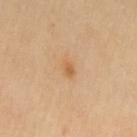On the left thigh.
Approximately 2 mm at its widest.
The subject is a female aged 68–72.
This image is a 15 mm lesion crop taken from a total-body photograph.
An algorithmic analysis of the crop reported a nevus-likeness score of about 35/100.
Captured under cross-polarized illumination.